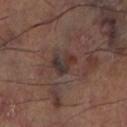Imaged during a routine full-body skin examination; the lesion was not biopsied and no histopathology is available. A region of skin cropped from a whole-body photographic capture, roughly 15 mm wide. Located on the left thigh.